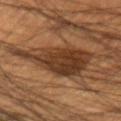Q: Was this lesion biopsied?
A: total-body-photography surveillance lesion; no biopsy
Q: What is the lesion's diameter?
A: ≈7.5 mm
Q: What is the anatomic site?
A: the left upper arm
Q: What is the imaging modality?
A: ~15 mm tile from a whole-body skin photo
Q: Who is the patient?
A: male, about 50 years old
Q: How was the tile lit?
A: cross-polarized illumination